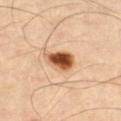workup — total-body-photography surveillance lesion; no biopsy | image source — total-body-photography crop, ~15 mm field of view | site — the left thigh.